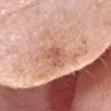Impression:
Imaged during a routine full-body skin examination; the lesion was not biopsied and no histopathology is available.